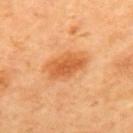Clinical impression:
Recorded during total-body skin imaging; not selected for excision or biopsy.
Background:
The patient is a male in their mid- to late 60s. The lesion is on the upper back. This image is a 15 mm lesion crop taken from a total-body photograph.